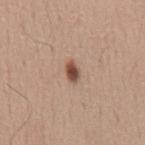Case summary:
• workup · imaged on a skin check; not biopsied
• illumination · white-light illumination
• diameter · ≈2.5 mm
• location · the chest
• image · ~15 mm crop, total-body skin-cancer survey
• patient · male, aged 58 to 62